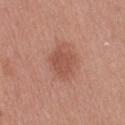{
  "biopsy_status": "not biopsied; imaged during a skin examination",
  "lighting": "white-light",
  "patient": {
    "sex": "female",
    "age_approx": 60
  },
  "automated_metrics": {
    "area_mm2_approx": 7.5,
    "eccentricity": 0.3,
    "shape_asymmetry": 0.35,
    "nevus_likeness_0_100": 60
  },
  "lesion_size": {
    "long_diameter_mm_approx": 3.0
  },
  "image": {
    "source": "total-body photography crop",
    "field_of_view_mm": 15
  },
  "site": "right thigh"
}